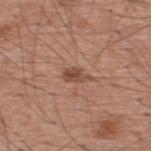lighting: white-light | diameter: ≈3 mm | subject: male, aged around 60 | image: total-body-photography crop, ~15 mm field of view | automated metrics: an area of roughly 3.5 mm², an eccentricity of roughly 0.85, and two-axis asymmetry of about 0.3; an average lesion color of about L≈47 a*≈21 b*≈27 (CIELAB) and a normalized border contrast of about 7.5; an automated nevus-likeness rating near 95 out of 100 and a lesion-detection confidence of about 100/100 | anatomic site: the upper back.